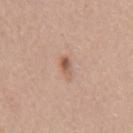<record>
<automated_metrics>
  <area_mm2_approx>3.5</area_mm2_approx>
  <nevus_likeness_0_100>85</nevus_likeness_0_100>
  <lesion_detection_confidence_0_100>100</lesion_detection_confidence_0_100>
</automated_metrics>
<image>
  <source>total-body photography crop</source>
  <field_of_view_mm>15</field_of_view_mm>
</image>
<lesion_size>
  <long_diameter_mm_approx>2.5</long_diameter_mm_approx>
</lesion_size>
<site>arm</site>
<patient>
  <sex>female</sex>
  <age_approx>25</age_approx>
</patient>
<lighting>white-light</lighting>
</record>